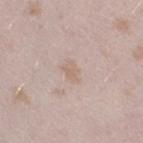Clinical impression:
No biopsy was performed on this lesion — it was imaged during a full skin examination and was not determined to be concerning.
Background:
Cropped from a total-body skin-imaging series; the visible field is about 15 mm. Imaged with white-light lighting. From the right thigh. Measured at roughly 3 mm in maximum diameter. A female patient aged approximately 25.